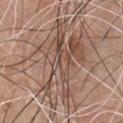  biopsy_status: not biopsied; imaged during a skin examination
  image:
    source: total-body photography crop
    field_of_view_mm: 15
  site: chest
  lighting: white-light
  patient:
    sex: male
    age_approx: 65